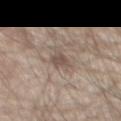Background:
Cropped from a total-body skin-imaging series; the visible field is about 15 mm. The tile uses white-light illumination. The lesion is on the chest. A male subject about 80 years old. Approximately 3 mm at its widest.